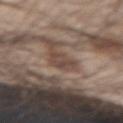Assessment:
The lesion was tiled from a total-body skin photograph and was not biopsied.
Context:
A male patient about 30 years old. A lesion tile, about 15 mm wide, cut from a 3D total-body photograph. From the left forearm.